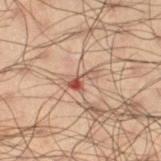Impression:
Imaged during a routine full-body skin examination; the lesion was not biopsied and no histopathology is available.
Background:
The lesion is on the right thigh. The lesion's longest dimension is about 3.5 mm. This is a cross-polarized tile. A lesion tile, about 15 mm wide, cut from a 3D total-body photograph. The subject is a male aged approximately 50.